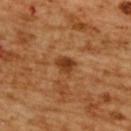Recorded during total-body skin imaging; not selected for excision or biopsy. A female patient, about 55 years old. A lesion tile, about 15 mm wide, cut from a 3D total-body photograph. From the back.